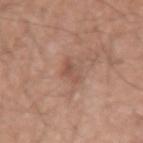The lesion was tiled from a total-body skin photograph and was not biopsied.
A roughly 15 mm field-of-view crop from a total-body skin photograph.
From the left upper arm.
The subject is a male approximately 55 years of age.